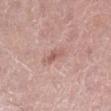The lesion was photographed on a routine skin check and not biopsied; there is no pathology result.
Cropped from a total-body skin-imaging series; the visible field is about 15 mm.
Measured at roughly 2.5 mm in maximum diameter.
The lesion is located on the right lower leg.
A male patient aged 53 to 57.